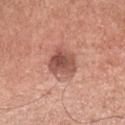follow-up = catalogued during a skin exam; not biopsied
diameter = ~4 mm (longest diameter)
imaging modality = total-body-photography crop, ~15 mm field of view
lighting = white-light illumination
patient = male, in their mid-50s
body site = the chest